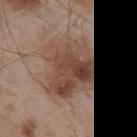Impression:
Part of a total-body skin-imaging series; this lesion was reviewed on a skin check and was not flagged for biopsy.
Context:
A 15 mm close-up extracted from a 3D total-body photography capture. Approximately 7 mm at its widest. The lesion is on the mid back. An algorithmic analysis of the crop reported about 11 CIELAB-L* units darker than the surrounding skin and a normalized lesion–skin contrast near 8.5. And it measured a peripheral color-asymmetry measure near 3. The patient is a male in their mid-50s. The tile uses white-light illumination.